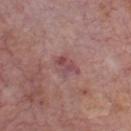Imaged during a routine full-body skin examination; the lesion was not biopsied and no histopathology is available.
A roughly 15 mm field-of-view crop from a total-body skin photograph.
A male subject in their mid-70s.
The lesion's longest dimension is about 3.5 mm.
This is a white-light tile.
Automated image analysis of the tile measured an area of roughly 5 mm², an outline eccentricity of about 0.75 (0 = round, 1 = elongated), and two-axis asymmetry of about 0.35.
From the chest.